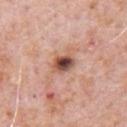patient=male, aged 78 to 82
body site=the chest
image-analysis metrics=an average lesion color of about L≈51 a*≈23 b*≈27 (CIELAB) and about 17 CIELAB-L* units darker than the surrounding skin; border irregularity of about 2 on a 0–10 scale, a color-variation rating of about 8.5/10, and a peripheral color-asymmetry measure near 2; an automated nevus-likeness rating near 85 out of 100
diameter=about 3 mm
acquisition=~15 mm crop, total-body skin-cancer survey